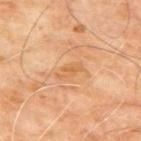Impression:
Part of a total-body skin-imaging series; this lesion was reviewed on a skin check and was not flagged for biopsy.
Background:
A 15 mm crop from a total-body photograph taken for skin-cancer surveillance. Approximately 3 mm at its widest. Imaged with cross-polarized lighting. On the back. The lesion-visualizer software estimated an area of roughly 3.5 mm², an eccentricity of roughly 0.9, and a shape-asymmetry score of about 0.35 (0 = symmetric). The analysis additionally found a color-variation rating of about 0.5/10 and radial color variation of about 0.5. The software also gave a detector confidence of about 100 out of 100 that the crop contains a lesion. The patient is a male roughly 65 years of age.